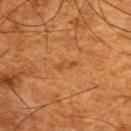Part of a total-body skin-imaging series; this lesion was reviewed on a skin check and was not flagged for biopsy.
This is a cross-polarized tile.
From the upper back.
The subject is a male aged around 65.
A lesion tile, about 15 mm wide, cut from a 3D total-body photograph.